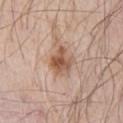Acquisition and patient details:
About 3.5 mm across. From the right upper arm. Captured under white-light illumination. This image is a 15 mm lesion crop taken from a total-body photograph. The lesion-visualizer software estimated a footprint of about 9 mm², a shape eccentricity near 0.5, and two-axis asymmetry of about 0.25. The analysis additionally found a lesion color around L≈56 a*≈19 b*≈30 in CIELAB and a normalized border contrast of about 8.5. The software also gave a border-irregularity index near 3.5/10, a within-lesion color-variation index near 5.5/10, and peripheral color asymmetry of about 2. And it measured an automated nevus-likeness rating near 60 out of 100 and a detector confidence of about 100 out of 100 that the crop contains a lesion. A male patient in their 70s.